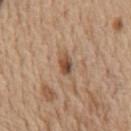Clinical impression: This lesion was catalogued during total-body skin photography and was not selected for biopsy. Image and clinical context: A region of skin cropped from a whole-body photographic capture, roughly 15 mm wide. A male patient, about 70 years old. On the chest.